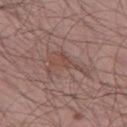Captured during whole-body skin photography for melanoma surveillance; the lesion was not biopsied.
Located on the left thigh.
This is a white-light tile.
A male subject aged around 55.
A 15 mm close-up tile from a total-body photography series done for melanoma screening.
An algorithmic analysis of the crop reported an area of roughly 10 mm² and a shape-asymmetry score of about 0.65 (0 = symmetric). The analysis additionally found a within-lesion color-variation index near 2.5/10 and peripheral color asymmetry of about 1.
Longest diameter approximately 5.5 mm.